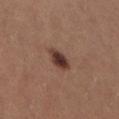| feature | finding |
|---|---|
| body site | the right thigh |
| patient | female, roughly 30 years of age |
| image source | 15 mm crop, total-body photography |
| image-analysis metrics | an area of roughly 4.5 mm², an outline eccentricity of about 0.8 (0 = round, 1 = elongated), and a shape-asymmetry score of about 0.2 (0 = symmetric); a lesion color around L≈36 a*≈19 b*≈23 in CIELAB, a lesion–skin lightness drop of about 14, and a normalized lesion–skin contrast near 12 |